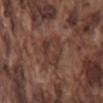  biopsy_status: not biopsied; imaged during a skin examination
  patient:
    sex: male
    age_approx: 75
  image:
    source: total-body photography crop
    field_of_view_mm: 15
  site: mid back